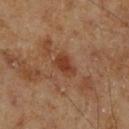Assessment: No biopsy was performed on this lesion — it was imaged during a full skin examination and was not determined to be concerning.